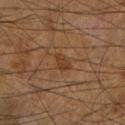This lesion was catalogued during total-body skin photography and was not selected for biopsy.
Approximately 2.5 mm at its widest.
Automated tile analysis of the lesion measured an area of roughly 3.5 mm² and two-axis asymmetry of about 0.25. The analysis additionally found a lesion color around L≈37 a*≈20 b*≈31 in CIELAB and about 7 CIELAB-L* units darker than the surrounding skin.
A 15 mm close-up tile from a total-body photography series done for melanoma screening.
From the left lower leg.
The tile uses cross-polarized illumination.
A male patient, about 65 years old.